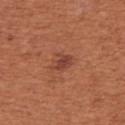Clinical impression:
No biopsy was performed on this lesion — it was imaged during a full skin examination and was not determined to be concerning.
Context:
The recorded lesion diameter is about 2.5 mm. The lesion is on the right upper arm. Cropped from a whole-body photographic skin survey; the tile spans about 15 mm. The lesion-visualizer software estimated a footprint of about 3.5 mm², an eccentricity of roughly 0.75, and a shape-asymmetry score of about 0.3 (0 = symmetric). And it measured a border-irregularity index near 3.5/10, a color-variation rating of about 1.5/10, and radial color variation of about 0.5. The software also gave a classifier nevus-likeness of about 35/100 and lesion-presence confidence of about 100/100. The patient is a female in their mid- to late 60s. Imaged with white-light lighting.